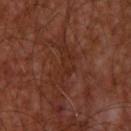Q: Was this lesion biopsied?
A: no biopsy performed (imaged during a skin exam)
Q: Who is the patient?
A: male, in their mid- to late 50s
Q: How large is the lesion?
A: ~5 mm (longest diameter)
Q: Lesion location?
A: the upper back
Q: What kind of image is this?
A: total-body-photography crop, ~15 mm field of view
Q: What did automated image analysis measure?
A: border irregularity of about 9 on a 0–10 scale, a within-lesion color-variation index near 2/10, and a peripheral color-asymmetry measure near 0.5